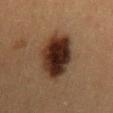{
  "biopsy_status": "not biopsied; imaged during a skin examination",
  "patient": {
    "sex": "female",
    "age_approx": 40
  },
  "site": "mid back",
  "image": {
    "source": "total-body photography crop",
    "field_of_view_mm": 15
  },
  "lesion_size": {
    "long_diameter_mm_approx": 7.5
  },
  "lighting": "cross-polarized"
}